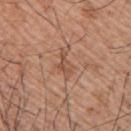  biopsy_status: not biopsied; imaged during a skin examination
  patient:
    sex: male
    age_approx: 55
  lighting: white-light
  automated_metrics:
    cielab_L: 51
    cielab_a: 21
    cielab_b: 31
    vs_skin_darker_L: 8.0
    vs_skin_contrast_norm: 6.0
  site: upper back
  image:
    source: total-body photography crop
    field_of_view_mm: 15
  lesion_size:
    long_diameter_mm_approx: 3.0